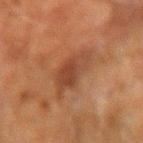follow-up: total-body-photography surveillance lesion; no biopsy
image: 15 mm crop, total-body photography
size: about 5 mm
anatomic site: the left arm
patient: male, aged around 60
tile lighting: cross-polarized